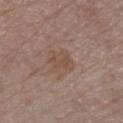Imaged during a routine full-body skin examination; the lesion was not biopsied and no histopathology is available.